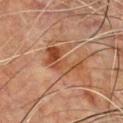biopsy status=no biopsy performed (imaged during a skin exam)